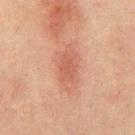No biopsy was performed on this lesion — it was imaged during a full skin examination and was not determined to be concerning. The subject is a male aged 63 to 67. Cropped from a total-body skin-imaging series; the visible field is about 15 mm. Located on the mid back. The tile uses cross-polarized illumination.